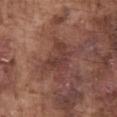– imaging modality: total-body-photography crop, ~15 mm field of view
– automated metrics: an average lesion color of about L≈39 a*≈22 b*≈23 (CIELAB), roughly 7 lightness units darker than nearby skin, and a normalized lesion–skin contrast near 6.5; a border-irregularity rating of about 6.5/10 and internal color variation of about 2.5 on a 0–10 scale
– tile lighting: white-light illumination
– site: the abdomen
– subject: male, aged 73–77
– lesion size: about 4 mm